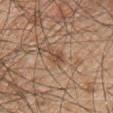<case>
<biopsy_status>not biopsied; imaged during a skin examination</biopsy_status>
<lesion_size>
  <long_diameter_mm_approx>3.0</long_diameter_mm_approx>
</lesion_size>
<site>chest</site>
<image>
  <source>total-body photography crop</source>
  <field_of_view_mm>15</field_of_view_mm>
</image>
<patient>
  <sex>male</sex>
  <age_approx>50</age_approx>
</patient>
<lighting>white-light</lighting>
<automated_metrics>
  <area_mm2_approx>3.5</area_mm2_approx>
  <eccentricity>0.85</eccentricity>
  <shape_asymmetry>0.4</shape_asymmetry>
  <vs_skin_darker_L>9.0</vs_skin_darker_L>
  <vs_skin_contrast_norm>6.5</vs_skin_contrast_norm>
  <border_irregularity_0_10>4.5</border_irregularity_0_10>
  <color_variation_0_10>3.0</color_variation_0_10>
  <peripheral_color_asymmetry>1.0</peripheral_color_asymmetry>
  <nevus_likeness_0_100>20</nevus_likeness_0_100>
</automated_metrics>
</case>